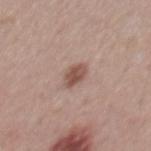site: mid back
lesion_size:
  long_diameter_mm_approx: 3.0
patient:
  sex: male
  age_approx: 55
lighting: white-light
image:
  source: total-body photography crop
  field_of_view_mm: 15